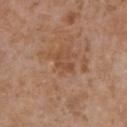– biopsy status — catalogued during a skin exam; not biopsied
– image — total-body-photography crop, ~15 mm field of view
– subject — female, aged 73–77
– body site — the front of the torso